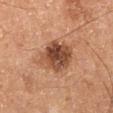No biopsy was performed on this lesion — it was imaged during a full skin examination and was not determined to be concerning. On the right lower leg. A male subject approximately 60 years of age. This is a cross-polarized tile. Automated image analysis of the tile measured an average lesion color of about L≈47 a*≈23 b*≈31 (CIELAB), a lesion–skin lightness drop of about 15, and a normalized border contrast of about 10.5. The software also gave border irregularity of about 2 on a 0–10 scale, a within-lesion color-variation index near 7.5/10, and peripheral color asymmetry of about 2.5. And it measured a classifier nevus-likeness of about 70/100 and a detector confidence of about 100 out of 100 that the crop contains a lesion. A 15 mm crop from a total-body photograph taken for skin-cancer surveillance. Measured at roughly 6 mm in maximum diameter.